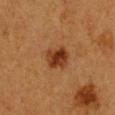Q: Was this lesion biopsied?
A: catalogued during a skin exam; not biopsied
Q: What kind of image is this?
A: total-body-photography crop, ~15 mm field of view
Q: What is the lesion's diameter?
A: ~3 mm (longest diameter)
Q: How was the tile lit?
A: cross-polarized illumination
Q: What is the anatomic site?
A: the chest
Q: What are the patient's age and sex?
A: female, aged 38–42